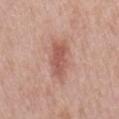Clinical impression: No biopsy was performed on this lesion — it was imaged during a full skin examination and was not determined to be concerning. Context: The tile uses white-light illumination. On the mid back. Cropped from a total-body skin-imaging series; the visible field is about 15 mm. A male subject aged approximately 80. Measured at roughly 5 mm in maximum diameter. An algorithmic analysis of the crop reported a lesion area of about 8.5 mm², an eccentricity of roughly 0.9, and a symmetry-axis asymmetry near 0.3. And it measured a lesion color around L≈55 a*≈24 b*≈26 in CIELAB, about 11 CIELAB-L* units darker than the surrounding skin, and a normalized lesion–skin contrast near 7. It also reported a nevus-likeness score of about 65/100 and lesion-presence confidence of about 100/100.